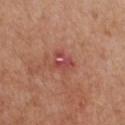Clinical impression:
The lesion was photographed on a routine skin check and not biopsied; there is no pathology result.
Context:
The total-body-photography lesion software estimated a mean CIELAB color near L≈48 a*≈31 b*≈26 and a lesion-to-skin contrast of about 6 (normalized; higher = more distinct). And it measured a border-irregularity rating of about 3/10 and peripheral color asymmetry of about 2.5. The lesion is on the chest. The subject is a male about 55 years old. About 3 mm across. The tile uses white-light illumination. Cropped from a total-body skin-imaging series; the visible field is about 15 mm.